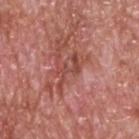biopsy_status: not biopsied; imaged during a skin examination
patient:
  sex: male
  age_approx: 60
image:
  source: total-body photography crop
  field_of_view_mm: 15
site: upper back
automated_metrics:
  border_irregularity_0_10: 10.0
  peripheral_color_asymmetry: 1.0
  nevus_likeness_0_100: 0
  lesion_detection_confidence_0_100: 60
lighting: white-light